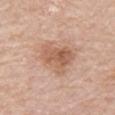Part of a total-body skin-imaging series; this lesion was reviewed on a skin check and was not flagged for biopsy. A female subject approximately 65 years of age. Captured under white-light illumination. Cropped from a whole-body photographic skin survey; the tile spans about 15 mm. On the arm. Approximately 4.5 mm at its widest.